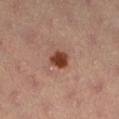Captured during whole-body skin photography for melanoma surveillance; the lesion was not biopsied.
A female subject, about 35 years old.
Longest diameter approximately 2.5 mm.
A region of skin cropped from a whole-body photographic capture, roughly 15 mm wide.
From the left lower leg.
Captured under cross-polarized illumination.